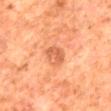Captured during whole-body skin photography for melanoma surveillance; the lesion was not biopsied. The lesion's longest dimension is about 3 mm. An algorithmic analysis of the crop reported a shape eccentricity near 0.7 and a symmetry-axis asymmetry near 0.2. The analysis additionally found a mean CIELAB color near L≈49 a*≈24 b*≈33, about 8 CIELAB-L* units darker than the surrounding skin, and a lesion-to-skin contrast of about 6.5 (normalized; higher = more distinct). The analysis additionally found a border-irregularity rating of about 2/10, a color-variation rating of about 3/10, and peripheral color asymmetry of about 1. The analysis additionally found a nevus-likeness score of about 10/100 and a lesion-detection confidence of about 100/100. A 15 mm crop from a total-body photograph taken for skin-cancer surveillance. The lesion is located on the mid back. A male patient about 80 years old. The tile uses cross-polarized illumination.